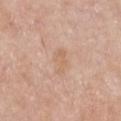The lesion was photographed on a routine skin check and not biopsied; there is no pathology result. An algorithmic analysis of the crop reported border irregularity of about 3.5 on a 0–10 scale, internal color variation of about 1.5 on a 0–10 scale, and a peripheral color-asymmetry measure near 0.5. The analysis additionally found a classifier nevus-likeness of about 0/100 and a detector confidence of about 100 out of 100 that the crop contains a lesion. A female patient, roughly 70 years of age. Imaged with white-light lighting. Cropped from a whole-body photographic skin survey; the tile spans about 15 mm. Located on the chest. Approximately 3.5 mm at its widest.